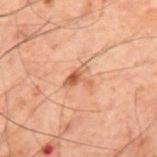This lesion was catalogued during total-body skin photography and was not selected for biopsy.
Located on the mid back.
The tile uses cross-polarized illumination.
The patient is a male roughly 60 years of age.
The total-body-photography lesion software estimated an area of roughly 4.5 mm², an outline eccentricity of about 0.55 (0 = round, 1 = elongated), and a shape-asymmetry score of about 0.55 (0 = symmetric). And it measured a lesion color around L≈46 a*≈20 b*≈29 in CIELAB, roughly 9 lightness units darker than nearby skin, and a normalized border contrast of about 7. The analysis additionally found a border-irregularity index near 6.5/10, a color-variation rating of about 2.5/10, and a peripheral color-asymmetry measure near 0.5. It also reported a nevus-likeness score of about 25/100 and a lesion-detection confidence of about 100/100.
The lesion's longest dimension is about 3 mm.
Cropped from a whole-body photographic skin survey; the tile spans about 15 mm.